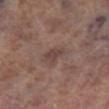notes = catalogued during a skin exam; not biopsied
location = the right lower leg
diameter = ≈3 mm
imaging modality = 15 mm crop, total-body photography
illumination = white-light illumination
subject = male, aged 68–72